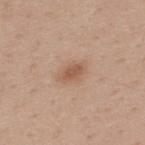follow-up: imaged on a skin check; not biopsied
tile lighting: white-light
image: total-body-photography crop, ~15 mm field of view
image-analysis metrics: a lesion area of about 4.5 mm², an outline eccentricity of about 0.8 (0 = round, 1 = elongated), and a shape-asymmetry score of about 0.2 (0 = symmetric); a mean CIELAB color near L≈56 a*≈20 b*≈31 and about 10 CIELAB-L* units darker than the surrounding skin; a detector confidence of about 100 out of 100 that the crop contains a lesion
patient: male, aged around 40
diameter: ~3 mm (longest diameter)
anatomic site: the upper back